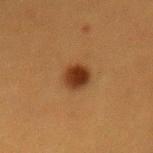Q: Was a biopsy performed?
A: imaged on a skin check; not biopsied
Q: Where on the body is the lesion?
A: the mid back
Q: What is the imaging modality?
A: ~15 mm crop, total-body skin-cancer survey
Q: How large is the lesion?
A: about 3 mm
Q: What are the patient's age and sex?
A: female, aged approximately 40
Q: How was the tile lit?
A: cross-polarized illumination
Q: What did automated image analysis measure?
A: an area of roughly 6 mm², a shape eccentricity near 0.65, and a symmetry-axis asymmetry near 0.15; a mean CIELAB color near L≈30 a*≈20 b*≈30 and roughly 12 lightness units darker than nearby skin; a classifier nevus-likeness of about 100/100 and lesion-presence confidence of about 100/100